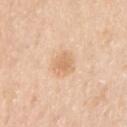Findings:
• workup · total-body-photography surveillance lesion; no biopsy
• patient · female, aged around 50
• site · the right upper arm
• image source · ~15 mm crop, total-body skin-cancer survey